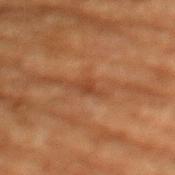Q: Was this lesion biopsied?
A: catalogued during a skin exam; not biopsied
Q: Illumination type?
A: cross-polarized
Q: Lesion size?
A: about 3.5 mm
Q: Patient demographics?
A: female, aged approximately 80
Q: Automated lesion metrics?
A: an area of roughly 3 mm², a shape eccentricity near 0.95, and two-axis asymmetry of about 0.3; a color-variation rating of about 0.5/10 and a peripheral color-asymmetry measure near 0; a classifier nevus-likeness of about 0/100 and a detector confidence of about 60 out of 100 that the crop contains a lesion
Q: Lesion location?
A: the chest
Q: What kind of image is this?
A: total-body-photography crop, ~15 mm field of view